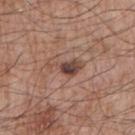A 15 mm crop from a total-body photograph taken for skin-cancer surveillance. The lesion is located on the right upper arm. A male subject, aged approximately 65.